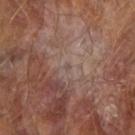The lesion was photographed on a routine skin check and not biopsied; there is no pathology result. The lesion-visualizer software estimated a lesion area of about 0.5 mm², a shape eccentricity near 0.8, and a symmetry-axis asymmetry near 0.3. The software also gave a lesion color around L≈47 a*≈15 b*≈19 in CIELAB. It also reported a classifier nevus-likeness of about 0/100. Located on the right forearm. The lesion's longest dimension is about 1 mm. A lesion tile, about 15 mm wide, cut from a 3D total-body photograph. A male patient aged 68 to 72.